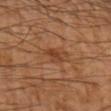biopsy status: catalogued during a skin exam; not biopsied | anatomic site: the left forearm | illumination: cross-polarized illumination | image: ~15 mm crop, total-body skin-cancer survey.